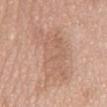This is a white-light tile.
A lesion tile, about 15 mm wide, cut from a 3D total-body photograph.
The subject is a female roughly 60 years of age.
The lesion-visualizer software estimated a lesion area of about 26 mm², a shape eccentricity near 0.9, and a shape-asymmetry score of about 0.25 (0 = symmetric). It also reported border irregularity of about 5.5 on a 0–10 scale, a color-variation rating of about 3/10, and radial color variation of about 1. It also reported a classifier nevus-likeness of about 0/100 and a detector confidence of about 95 out of 100 that the crop contains a lesion.
The lesion is on the mid back.
The recorded lesion diameter is about 8.5 mm.